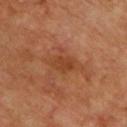Q: What did automated image analysis measure?
A: a footprint of about 6 mm² and a symmetry-axis asymmetry near 0.2; about 7 CIELAB-L* units darker than the surrounding skin and a normalized lesion–skin contrast near 6; an automated nevus-likeness rating near 0 out of 100 and lesion-presence confidence of about 100/100
Q: Patient demographics?
A: male, roughly 65 years of age
Q: What is the imaging modality?
A: total-body-photography crop, ~15 mm field of view
Q: Where on the body is the lesion?
A: the chest
Q: How was the tile lit?
A: cross-polarized illumination